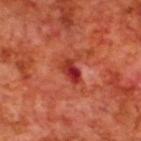This lesion was catalogued during total-body skin photography and was not selected for biopsy. On the back. A 15 mm close-up tile from a total-body photography series done for melanoma screening. A male subject aged around 70.